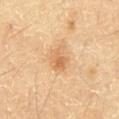Q: Was this lesion biopsied?
A: imaged on a skin check; not biopsied
Q: Patient demographics?
A: male, aged approximately 60
Q: What is the imaging modality?
A: ~15 mm tile from a whole-body skin photo